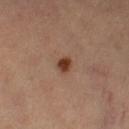{"biopsy_status": "not biopsied; imaged during a skin examination", "lighting": "cross-polarized", "lesion_size": {"long_diameter_mm_approx": 2.5}, "patient": {"sex": "female", "age_approx": 65}, "image": {"source": "total-body photography crop", "field_of_view_mm": 15}, "site": "left lower leg"}